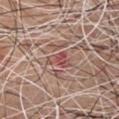Clinical impression: Recorded during total-body skin imaging; not selected for excision or biopsy. Image and clinical context: Cropped from a whole-body photographic skin survey; the tile spans about 15 mm. A male patient roughly 65 years of age. The lesion is located on the chest. The tile uses white-light illumination. Automated image analysis of the tile measured an average lesion color of about L≈50 a*≈25 b*≈24 (CIELAB). The analysis additionally found a classifier nevus-likeness of about 0/100 and a detector confidence of about 85 out of 100 that the crop contains a lesion.